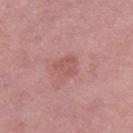Imaged during a routine full-body skin examination; the lesion was not biopsied and no histopathology is available. About 3 mm across. A 15 mm close-up extracted from a 3D total-body photography capture. On the right thigh. The patient is a female aged around 40. Imaged with white-light lighting.